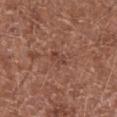Captured during whole-body skin photography for melanoma surveillance; the lesion was not biopsied.
The subject is a male roughly 65 years of age.
The lesion's longest dimension is about 2.5 mm.
From the abdomen.
This image is a 15 mm lesion crop taken from a total-body photograph.
This is a white-light tile.